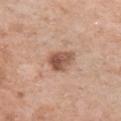– workup — imaged on a skin check; not biopsied
– lighting — white-light illumination
– body site — the chest
– subject — female, approximately 50 years of age
– acquisition — 15 mm crop, total-body photography